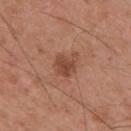Captured during whole-body skin photography for melanoma surveillance; the lesion was not biopsied.
Located on the mid back.
Cropped from a whole-body photographic skin survey; the tile spans about 15 mm.
A male subject, about 55 years old.